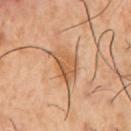The lesion was tiled from a total-body skin photograph and was not biopsied. Captured under cross-polarized illumination. Located on the chest. The patient is a male aged 53 to 57. A 15 mm close-up extracted from a 3D total-body photography capture. Approximately 4.5 mm at its widest.